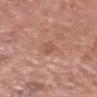Q: Where on the body is the lesion?
A: the head or neck
Q: What are the patient's age and sex?
A: male, approximately 60 years of age
Q: How was this image acquired?
A: 15 mm crop, total-body photography